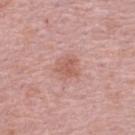biopsy status: imaged on a skin check; not biopsied
anatomic site: the upper back
acquisition: 15 mm crop, total-body photography
lighting: white-light illumination
subject: female, in their mid- to late 60s